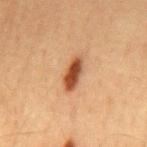Imaged during a routine full-body skin examination; the lesion was not biopsied and no histopathology is available.
A male patient in their mid-60s.
A 15 mm close-up tile from a total-body photography series done for melanoma screening.
The lesion is on the back.